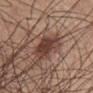From the chest. A roughly 15 mm field-of-view crop from a total-body skin photograph. The tile uses white-light illumination. A male subject aged around 65. About 4 mm across.